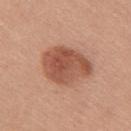Part of a total-body skin-imaging series; this lesion was reviewed on a skin check and was not flagged for biopsy. A female subject aged 33 to 37. Measured at roughly 6 mm in maximum diameter. The lesion is located on the right upper arm. A roughly 15 mm field-of-view crop from a total-body skin photograph. The tile uses white-light illumination.